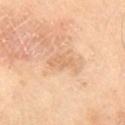Findings:
- follow-up · total-body-photography surveillance lesion; no biopsy
- acquisition · ~15 mm tile from a whole-body skin photo
- subject · male, in their mid-60s
- lighting · cross-polarized illumination
- body site · the right thigh